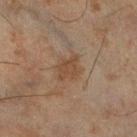Recorded during total-body skin imaging; not selected for excision or biopsy.
A male patient, aged approximately 45.
Captured under cross-polarized illumination.
On the right lower leg.
A roughly 15 mm field-of-view crop from a total-body skin photograph.